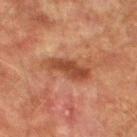Findings:
* biopsy status: total-body-photography surveillance lesion; no biopsy
* size: ~5.5 mm (longest diameter)
* location: the left lower leg
* acquisition: 15 mm crop, total-body photography
* patient: male, aged around 75
* illumination: cross-polarized
* automated metrics: a lesion area of about 9.5 mm², an eccentricity of roughly 0.9, and two-axis asymmetry of about 0.3; a border-irregularity index near 4/10, a color-variation rating of about 3/10, and a peripheral color-asymmetry measure near 1; a classifier nevus-likeness of about 0/100 and a detector confidence of about 100 out of 100 that the crop contains a lesion